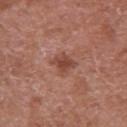- follow-up — total-body-photography surveillance lesion; no biopsy
- patient — male, aged 63–67
- body site — the right upper arm
- image source — ~15 mm crop, total-body skin-cancer survey